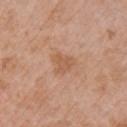<lesion>
<biopsy_status>not biopsied; imaged during a skin examination</biopsy_status>
<lighting>white-light</lighting>
<patient>
  <sex>female</sex>
  <age_approx>40</age_approx>
</patient>
<site>chest</site>
<image>
  <source>total-body photography crop</source>
  <field_of_view_mm>15</field_of_view_mm>
</image>
</lesion>